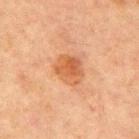The lesion was photographed on a routine skin check and not biopsied; there is no pathology result.
A male subject, about 65 years old.
A 15 mm close-up tile from a total-body photography series done for melanoma screening.
On the chest.
Captured under cross-polarized illumination.
The lesion's longest dimension is about 3.5 mm.